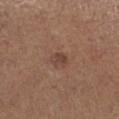workup: no biopsy performed (imaged during a skin exam) | tile lighting: white-light illumination | image source: 15 mm crop, total-body photography | TBP lesion metrics: border irregularity of about 1.5 on a 0–10 scale, a within-lesion color-variation index near 3/10, and a peripheral color-asymmetry measure near 1; a nevus-likeness score of about 40/100 | site: the left lower leg | subject: male, in their mid- to late 60s.